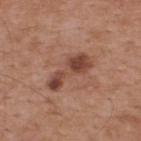Imaged during a routine full-body skin examination; the lesion was not biopsied and no histopathology is available. The patient is a male aged around 55. The lesion's longest dimension is about 5.5 mm. From the upper back. A lesion tile, about 15 mm wide, cut from a 3D total-body photograph.